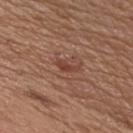biopsy status — catalogued during a skin exam; not biopsied | lesion size — ≈3 mm | lighting — white-light | location — the chest | subject — male, about 55 years old | acquisition — total-body-photography crop, ~15 mm field of view.